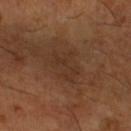workup — total-body-photography surveillance lesion; no biopsy
site — the left lower leg
subject — male, about 65 years old
image source — 15 mm crop, total-body photography
lighting — cross-polarized
diameter — ~4 mm (longest diameter)
automated metrics — an area of roughly 7 mm²; border irregularity of about 5.5 on a 0–10 scale, a within-lesion color-variation index near 1.5/10, and a peripheral color-asymmetry measure near 0.5; a nevus-likeness score of about 0/100 and a lesion-detection confidence of about 100/100